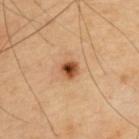workup=catalogued during a skin exam; not biopsied
image source=total-body-photography crop, ~15 mm field of view
site=the upper back
patient=male, aged approximately 65